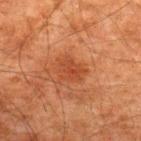| field | value |
|---|---|
| workup | total-body-photography surveillance lesion; no biopsy |
| body site | the upper back |
| subject | male, in their 60s |
| acquisition | 15 mm crop, total-body photography |
| lesion diameter | about 3.5 mm |
| automated lesion analysis | a shape eccentricity near 0.65 and two-axis asymmetry of about 0.3; border irregularity of about 3 on a 0–10 scale and peripheral color asymmetry of about 1 |
| illumination | cross-polarized illumination |